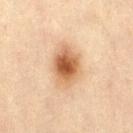| field | value |
|---|---|
| workup | catalogued during a skin exam; not biopsied |
| site | the left thigh |
| acquisition | ~15 mm tile from a whole-body skin photo |
| subject | female, aged 43–47 |
| diameter | ≈5 mm |
| automated lesion analysis | a footprint of about 12 mm² and an eccentricity of roughly 0.7; a mean CIELAB color near L≈53 a*≈20 b*≈33, a lesion–skin lightness drop of about 14, and a lesion-to-skin contrast of about 10 (normalized; higher = more distinct); internal color variation of about 6 on a 0–10 scale and a peripheral color-asymmetry measure near 1; a nevus-likeness score of about 100/100 and a detector confidence of about 100 out of 100 that the crop contains a lesion |